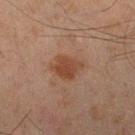This is a cross-polarized tile.
The lesion is on the right lower leg.
The patient is a male approximately 45 years of age.
This image is a 15 mm lesion crop taken from a total-body photograph.